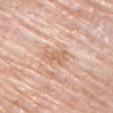Clinical impression: This lesion was catalogued during total-body skin photography and was not selected for biopsy. Context: The lesion is on the left upper arm. A female subject, aged 63 to 67. A 15 mm crop from a total-body photograph taken for skin-cancer surveillance. An algorithmic analysis of the crop reported a lesion area of about 4.5 mm² and an outline eccentricity of about 0.85 (0 = round, 1 = elongated). It also reported about 9 CIELAB-L* units darker than the surrounding skin. And it measured a classifier nevus-likeness of about 0/100 and lesion-presence confidence of about 90/100. Longest diameter approximately 3.5 mm.